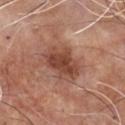Q: Was a biopsy performed?
A: catalogued during a skin exam; not biopsied
Q: What is the imaging modality?
A: total-body-photography crop, ~15 mm field of view
Q: Lesion location?
A: the front of the torso
Q: What are the patient's age and sex?
A: male, aged around 65